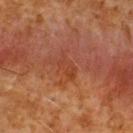Q: What lighting was used for the tile?
A: cross-polarized illumination
Q: What is the lesion's diameter?
A: about 3.5 mm
Q: Where on the body is the lesion?
A: the upper back
Q: How was this image acquired?
A: 15 mm crop, total-body photography
Q: Patient demographics?
A: male, aged around 60
Q: Automated lesion metrics?
A: a lesion area of about 5 mm²; roughly 5 lightness units darker than nearby skin and a normalized border contrast of about 5